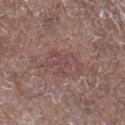tile lighting: white-light illumination
image: total-body-photography crop, ~15 mm field of view
image-analysis metrics: about 6 CIELAB-L* units darker than the surrounding skin and a lesion-to-skin contrast of about 4.5 (normalized; higher = more distinct); a classifier nevus-likeness of about 0/100 and a detector confidence of about 100 out of 100 that the crop contains a lesion
body site: the leg
lesion size: ≈4.5 mm
subject: male, aged 68–72